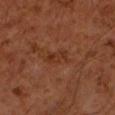Q: Was a biopsy performed?
A: total-body-photography surveillance lesion; no biopsy
Q: Automated lesion metrics?
A: an eccentricity of roughly 0.85 and a symmetry-axis asymmetry near 0.3; a mean CIELAB color near L≈30 a*≈23 b*≈29, about 6 CIELAB-L* units darker than the surrounding skin, and a normalized lesion–skin contrast near 5.5; a nevus-likeness score of about 0/100 and lesion-presence confidence of about 100/100
Q: How large is the lesion?
A: ≈3 mm
Q: What is the imaging modality?
A: ~15 mm tile from a whole-body skin photo
Q: Lesion location?
A: the left forearm
Q: Who is the patient?
A: male, aged around 60